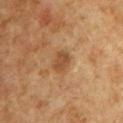biopsy_status: not biopsied; imaged during a skin examination
patient:
  sex: male
  age_approx: 60
lesion_size:
  long_diameter_mm_approx: 2.5
automated_metrics:
  cielab_L: 39
  cielab_a: 18
  cielab_b: 31
  vs_skin_darker_L: 8.0
  vs_skin_contrast_norm: 7.0
  nevus_likeness_0_100: 10
  lesion_detection_confidence_0_100: 100
lighting: cross-polarized
site: chest
image:
  source: total-body photography crop
  field_of_view_mm: 15